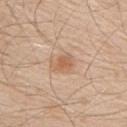{
  "biopsy_status": "not biopsied; imaged during a skin examination"
}